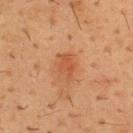Assessment:
Part of a total-body skin-imaging series; this lesion was reviewed on a skin check and was not flagged for biopsy.
Context:
Longest diameter approximately 3 mm. A male patient, aged around 55. Cropped from a total-body skin-imaging series; the visible field is about 15 mm. An algorithmic analysis of the crop reported a lesion area of about 4 mm² and an eccentricity of roughly 0.75. It also reported a mean CIELAB color near L≈45 a*≈24 b*≈34, roughly 7 lightness units darker than nearby skin, and a lesion-to-skin contrast of about 6 (normalized; higher = more distinct). And it measured an automated nevus-likeness rating near 25 out of 100 and a detector confidence of about 100 out of 100 that the crop contains a lesion. Imaged with cross-polarized lighting. From the chest.